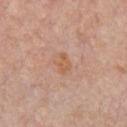<record>
<biopsy_status>not biopsied; imaged during a skin examination</biopsy_status>
<image>
  <source>total-body photography crop</source>
  <field_of_view_mm>15</field_of_view_mm>
</image>
<patient>
  <sex>male</sex>
  <age_approx>60</age_approx>
</patient>
<lesion_size>
  <long_diameter_mm_approx>2.5</long_diameter_mm_approx>
</lesion_size>
<site>chest</site>
<automated_metrics>
  <area_mm2_approx>3.5</area_mm2_approx>
  <eccentricity>0.7</eccentricity>
  <shape_asymmetry>0.25</shape_asymmetry>
  <cielab_L>59</cielab_L>
  <cielab_a>21</cielab_a>
  <cielab_b>34</cielab_b>
  <border_irregularity_0_10>2.0</border_irregularity_0_10>
  <color_variation_0_10>1.5</color_variation_0_10>
  <peripheral_color_asymmetry>0.5</peripheral_color_asymmetry>
</automated_metrics>
<lighting>white-light</lighting>
</record>